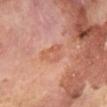Assessment:
Imaged during a routine full-body skin examination; the lesion was not biopsied and no histopathology is available.
Image and clinical context:
A male patient, aged 68–72. Approximately 3.5 mm at its widest. Automated tile analysis of the lesion measured an eccentricity of roughly 0.65 and two-axis asymmetry of about 0.45. And it measured a normalized lesion–skin contrast near 5. The software also gave a lesion-detection confidence of about 100/100. A lesion tile, about 15 mm wide, cut from a 3D total-body photograph. Located on the left lower leg.